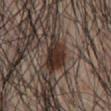follow-up: no biopsy performed (imaged during a skin exam) | imaging modality: ~15 mm tile from a whole-body skin photo | automated lesion analysis: a lesion area of about 10 mm², an outline eccentricity of about 0.8 (0 = round, 1 = elongated), and a shape-asymmetry score of about 0.3 (0 = symmetric); a lesion color around L≈30 a*≈14 b*≈20 in CIELAB and a lesion-to-skin contrast of about 13 (normalized; higher = more distinct); border irregularity of about 3.5 on a 0–10 scale, a color-variation rating of about 5.5/10, and radial color variation of about 2 | subject: male, aged 53 to 57 | site: the chest | lesion diameter: ~5 mm (longest diameter) | illumination: white-light.